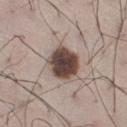workup: catalogued during a skin exam; not biopsied
diameter: ≈4 mm
image-analysis metrics: an outline eccentricity of about 0.4 (0 = round, 1 = elongated) and two-axis asymmetry of about 0.15; a lesion color around L≈43 a*≈15 b*≈20 in CIELAB and about 21 CIELAB-L* units darker than the surrounding skin; a border-irregularity rating of about 1.5/10 and a within-lesion color-variation index near 4.5/10; a nevus-likeness score of about 45/100
imaging modality: 15 mm crop, total-body photography
tile lighting: white-light
subject: male, in their mid- to late 60s
body site: the right thigh